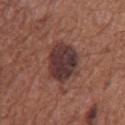Q: Was this lesion biopsied?
A: no biopsy performed (imaged during a skin exam)
Q: What kind of image is this?
A: 15 mm crop, total-body photography
Q: Lesion size?
A: about 5 mm
Q: Who is the patient?
A: male, aged around 75
Q: Where on the body is the lesion?
A: the front of the torso